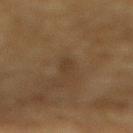Clinical impression: Recorded during total-body skin imaging; not selected for excision or biopsy. Image and clinical context: Cropped from a total-body skin-imaging series; the visible field is about 15 mm. The lesion is on the mid back. The patient is a male aged 83 or older.